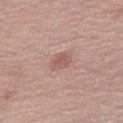This lesion was catalogued during total-body skin photography and was not selected for biopsy. A female subject, roughly 55 years of age. This is a white-light tile. An algorithmic analysis of the crop reported border irregularity of about 2.5 on a 0–10 scale, a within-lesion color-variation index near 1.5/10, and radial color variation of about 0.5. Cropped from a total-body skin-imaging series; the visible field is about 15 mm. The lesion is located on the right thigh. The recorded lesion diameter is about 2.5 mm.